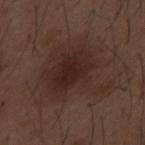Part of a total-body skin-imaging series; this lesion was reviewed on a skin check and was not flagged for biopsy. The subject is a male aged 48–52. A 15 mm crop from a total-body photograph taken for skin-cancer surveillance. From the back.